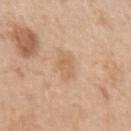Clinical impression:
Recorded during total-body skin imaging; not selected for excision or biopsy.
Image and clinical context:
The lesion is on the left upper arm. A male patient, in their mid- to late 50s. This is a white-light tile. Approximately 3.5 mm at its widest. Automated tile analysis of the lesion measured a lesion area of about 5.5 mm², a shape eccentricity near 0.8, and two-axis asymmetry of about 0.25. The analysis additionally found a border-irregularity index near 3/10, internal color variation of about 2 on a 0–10 scale, and radial color variation of about 0.5. A 15 mm close-up extracted from a 3D total-body photography capture.